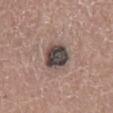Clinical impression: Recorded during total-body skin imaging; not selected for excision or biopsy. Acquisition and patient details: Approximately 3.5 mm at its widest. Captured under white-light illumination. A lesion tile, about 15 mm wide, cut from a 3D total-body photograph. The subject is a male approximately 55 years of age. The lesion-visualizer software estimated a lesion area of about 10 mm² and an eccentricity of roughly 0.4. The analysis additionally found a border-irregularity index near 1.5/10 and a peripheral color-asymmetry measure near 2.